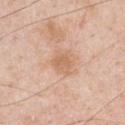Imaged during a routine full-body skin examination; the lesion was not biopsied and no histopathology is available.
A male subject aged 48 to 52.
Cropped from a total-body skin-imaging series; the visible field is about 15 mm.
The total-body-photography lesion software estimated a border-irregularity index near 2.5/10, internal color variation of about 2 on a 0–10 scale, and a peripheral color-asymmetry measure near 0.5. And it measured an automated nevus-likeness rating near 0 out of 100 and a detector confidence of about 100 out of 100 that the crop contains a lesion.
On the chest.
About 3 mm across.